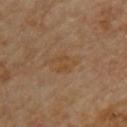<lesion>
  <image>
    <source>total-body photography crop</source>
    <field_of_view_mm>15</field_of_view_mm>
  </image>
  <patient>
    <sex>male</sex>
    <age_approx>85+</age_approx>
  </patient>
  <site>upper back</site>
</lesion>